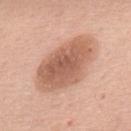Clinical summary: Measured at roughly 8.5 mm in maximum diameter. This image is a 15 mm lesion crop taken from a total-body photograph. The tile uses white-light illumination. The subject is a male aged around 75. Automated image analysis of the tile measured a footprint of about 28 mm², an outline eccentricity of about 0.85 (0 = round, 1 = elongated), and a symmetry-axis asymmetry near 0.15. And it measured a mean CIELAB color near L≈59 a*≈22 b*≈31, about 13 CIELAB-L* units darker than the surrounding skin, and a lesion-to-skin contrast of about 8 (normalized; higher = more distinct). The analysis additionally found a classifier nevus-likeness of about 10/100 and lesion-presence confidence of about 100/100. From the upper back.